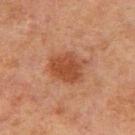Captured during whole-body skin photography for melanoma surveillance; the lesion was not biopsied. The lesion is located on the right upper arm. Captured under cross-polarized illumination. Cropped from a total-body skin-imaging series; the visible field is about 15 mm. The subject is a male roughly 70 years of age.